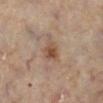Impression: No biopsy was performed on this lesion — it was imaged during a full skin examination and was not determined to be concerning. Image and clinical context: On the left lower leg. The subject is a female roughly 60 years of age. The tile uses cross-polarized illumination. About 2.5 mm across. A region of skin cropped from a whole-body photographic capture, roughly 15 mm wide.